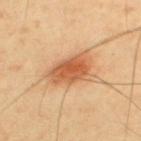Part of a total-body skin-imaging series; this lesion was reviewed on a skin check and was not flagged for biopsy.
Captured under cross-polarized illumination.
A male patient, aged approximately 65.
Measured at roughly 5 mm in maximum diameter.
A 15 mm close-up extracted from a 3D total-body photography capture.
Located on the back.
Automated tile analysis of the lesion measured a mean CIELAB color near L≈59 a*≈25 b*≈39, a lesion–skin lightness drop of about 13, and a normalized lesion–skin contrast near 8.5. It also reported a classifier nevus-likeness of about 100/100 and lesion-presence confidence of about 100/100.